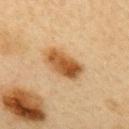follow-up: total-body-photography surveillance lesion; no biopsy | imaging modality: ~15 mm crop, total-body skin-cancer survey | size: ≈5 mm | body site: the upper back | illumination: cross-polarized | TBP lesion metrics: a lesion area of about 11 mm², an outline eccentricity of about 0.85 (0 = round, 1 = elongated), and two-axis asymmetry of about 0.2; about 13 CIELAB-L* units darker than the surrounding skin and a normalized lesion–skin contrast near 10.5; an automated nevus-likeness rating near 100 out of 100 and a lesion-detection confidence of about 100/100 | subject: female, aged 43 to 47.